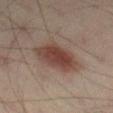Context:
A 15 mm close-up extracted from a 3D total-body photography capture. A male subject, roughly 40 years of age. On the left thigh.